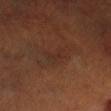Case summary:
– notes — catalogued during a skin exam; not biopsied
– tile lighting — cross-polarized
– subject — male, roughly 50 years of age
– image source — 15 mm crop, total-body photography
– body site — the right lower leg
– size — ≈2.5 mm
– image-analysis metrics — a footprint of about 3.5 mm² and a symmetry-axis asymmetry near 0.25; roughly 4 lightness units darker than nearby skin; internal color variation of about 1.5 on a 0–10 scale and radial color variation of about 0.5; lesion-presence confidence of about 65/100